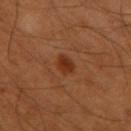Case summary:
• image source · 15 mm crop, total-body photography
• site · the left thigh
• patient · male, aged 53 to 57
• lighting · cross-polarized
• diameter · ~2.5 mm (longest diameter)